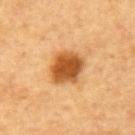<lesion>
  <biopsy_status>not biopsied; imaged during a skin examination</biopsy_status>
  <patient>
    <sex>male</sex>
    <age_approx>65</age_approx>
  </patient>
  <site>mid back</site>
  <image>
    <source>total-body photography crop</source>
    <field_of_view_mm>15</field_of_view_mm>
  </image>
</lesion>